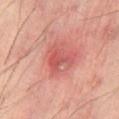  biopsy_status: not biopsied; imaged during a skin examination
  lighting: cross-polarized
  patient:
    sex: male
    age_approx: 60
  image:
    source: total-body photography crop
    field_of_view_mm: 15
  site: abdomen
  lesion_size:
    long_diameter_mm_approx: 4.0